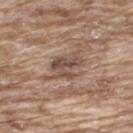biopsy status: total-body-photography surveillance lesion; no biopsy